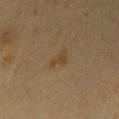{
  "biopsy_status": "not biopsied; imaged during a skin examination",
  "image": {
    "source": "total-body photography crop",
    "field_of_view_mm": 15
  },
  "site": "chest",
  "automated_metrics": {
    "area_mm2_approx": 3.5,
    "eccentricity": 0.8,
    "shape_asymmetry": 0.5,
    "border_irregularity_0_10": 5.0,
    "color_variation_0_10": 1.0,
    "peripheral_color_asymmetry": 0.5,
    "nevus_likeness_0_100": 30,
    "lesion_detection_confidence_0_100": 100
  },
  "patient": {
    "sex": "male",
    "age_approx": 60
  },
  "lesion_size": {
    "long_diameter_mm_approx": 3.0
  },
  "lighting": "cross-polarized"
}